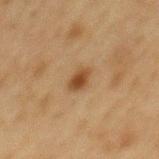| key | value |
|---|---|
| notes | no biopsy performed (imaged during a skin exam) |
| lesion size | ~3 mm (longest diameter) |
| illumination | cross-polarized illumination |
| location | the mid back |
| image source | ~15 mm crop, total-body skin-cancer survey |
| subject | male, aged 73 to 77 |